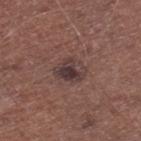Imaged during a routine full-body skin examination; the lesion was not biopsied and no histopathology is available.
The tile uses white-light illumination.
Automated tile analysis of the lesion measured a footprint of about 8 mm², an outline eccentricity of about 0.6 (0 = round, 1 = elongated), and a symmetry-axis asymmetry near 0.2. It also reported a border-irregularity rating of about 2/10 and a color-variation rating of about 7.5/10.
From the right lower leg.
Approximately 3.5 mm at its widest.
A male patient, roughly 65 years of age.
Cropped from a total-body skin-imaging series; the visible field is about 15 mm.